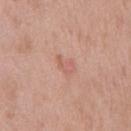Impression:
Captured during whole-body skin photography for melanoma surveillance; the lesion was not biopsied.
Background:
The lesion's longest dimension is about 2.5 mm. Cropped from a whole-body photographic skin survey; the tile spans about 15 mm. A male subject, aged 48 to 52. Captured under white-light illumination. The lesion is on the chest.